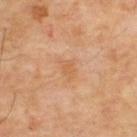Cropped from a whole-body photographic skin survey; the tile spans about 15 mm. A male patient aged around 55. Imaged with cross-polarized lighting. Longest diameter approximately 2.5 mm. The lesion is on the upper back.